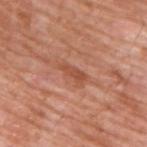The lesion was photographed on a routine skin check and not biopsied; there is no pathology result.
Imaged with white-light lighting.
Automated tile analysis of the lesion measured an average lesion color of about L≈51 a*≈27 b*≈33 (CIELAB) and a normalized lesion–skin contrast near 6.5. The analysis additionally found internal color variation of about 1 on a 0–10 scale and radial color variation of about 0. It also reported a nevus-likeness score of about 0/100 and lesion-presence confidence of about 95/100.
A male patient, aged around 60.
This image is a 15 mm lesion crop taken from a total-body photograph.
The recorded lesion diameter is about 3.5 mm.
On the upper back.